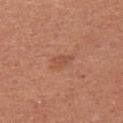This lesion was catalogued during total-body skin photography and was not selected for biopsy. The patient is a female aged 23–27. Approximately 2.5 mm at its widest. On the left upper arm. A close-up tile cropped from a whole-body skin photograph, about 15 mm across.